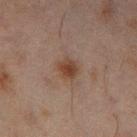| key | value |
|---|---|
| workup | no biopsy performed (imaged during a skin exam) |
| location | the left thigh |
| acquisition | ~15 mm crop, total-body skin-cancer survey |
| image-analysis metrics | a lesion area of about 4.5 mm², an outline eccentricity of about 0.55 (0 = round, 1 = elongated), and a symmetry-axis asymmetry near 0.15; a normalized lesion–skin contrast near 8.5 |
| subject | male, aged approximately 45 |
| tile lighting | cross-polarized illumination |
| size | ~2.5 mm (longest diameter) |